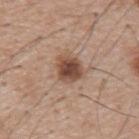biopsy status = no biopsy performed (imaged during a skin exam) | subject = male, aged around 55 | image = ~15 mm crop, total-body skin-cancer survey.